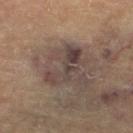Imaged during a routine full-body skin examination; the lesion was not biopsied and no histopathology is available. Automated tile analysis of the lesion measured an average lesion color of about L≈34 a*≈12 b*≈16 (CIELAB), about 8 CIELAB-L* units darker than the surrounding skin, and a lesion-to-skin contrast of about 8 (normalized; higher = more distinct). The analysis additionally found a color-variation rating of about 5.5/10. Located on the left lower leg. A female subject aged approximately 80. A 15 mm crop from a total-body photograph taken for skin-cancer surveillance. About 5 mm across. Imaged with cross-polarized lighting.